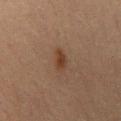Recorded during total-body skin imaging; not selected for excision or biopsy. A lesion tile, about 15 mm wide, cut from a 3D total-body photograph. The lesion is on the mid back. The subject is a male aged around 70.